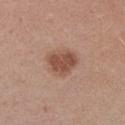<lesion>
  <biopsy_status>not biopsied; imaged during a skin examination</biopsy_status>
  <patient>
    <sex>male</sex>
    <age_approx>25</age_approx>
  </patient>
  <lighting>white-light</lighting>
  <site>left upper arm</site>
  <image>
    <source>total-body photography crop</source>
    <field_of_view_mm>15</field_of_view_mm>
  </image>
</lesion>